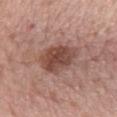Impression:
No biopsy was performed on this lesion — it was imaged during a full skin examination and was not determined to be concerning.
Acquisition and patient details:
This image is a 15 mm lesion crop taken from a total-body photograph. The lesion is on the mid back. Captured under white-light illumination. A female patient, approximately 65 years of age. Longest diameter approximately 5 mm.